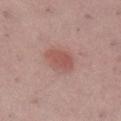Q: What kind of image is this?
A: ~15 mm crop, total-body skin-cancer survey
Q: Illumination type?
A: white-light
Q: Who is the patient?
A: female, aged around 55
Q: What is the lesion's diameter?
A: ~4 mm (longest diameter)
Q: Lesion location?
A: the right lower leg